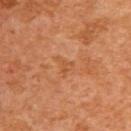imaging modality=~15 mm crop, total-body skin-cancer survey; size=~2.5 mm (longest diameter); site=the upper back; automated lesion analysis=border irregularity of about 5.5 on a 0–10 scale and internal color variation of about 1 on a 0–10 scale; subject=male, aged approximately 60.